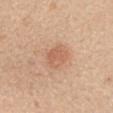The lesion was photographed on a routine skin check and not biopsied; there is no pathology result. The lesion's longest dimension is about 3 mm. A female subject aged 38 to 42. Cropped from a total-body skin-imaging series; the visible field is about 15 mm. Located on the mid back.